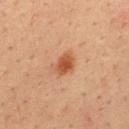follow-up = imaged on a skin check; not biopsied
size = ≈2.5 mm
automated metrics = a footprint of about 4.5 mm², a shape eccentricity near 0.65, and two-axis asymmetry of about 0.2; a lesion color around L≈49 a*≈25 b*≈36 in CIELAB and a normalized lesion–skin contrast near 9; a border-irregularity index near 2/10, a within-lesion color-variation index near 2.5/10, and a peripheral color-asymmetry measure near 1; a classifier nevus-likeness of about 100/100 and a lesion-detection confidence of about 100/100
acquisition = total-body-photography crop, ~15 mm field of view
anatomic site = the upper back
subject = male, roughly 35 years of age
lighting = cross-polarized